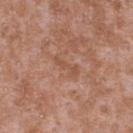Imaged during a routine full-body skin examination; the lesion was not biopsied and no histopathology is available. From the upper back. The subject is a male aged 43–47. About 3.5 mm across. A lesion tile, about 15 mm wide, cut from a 3D total-body photograph.